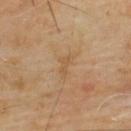Assessment:
The lesion was tiled from a total-body skin photograph and was not biopsied.
Context:
A male patient roughly 65 years of age. The lesion is on the upper back. A lesion tile, about 15 mm wide, cut from a 3D total-body photograph.